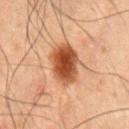notes = imaged on a skin check; not biopsied
image-analysis metrics = a footprint of about 14 mm², an eccentricity of roughly 0.7, and a symmetry-axis asymmetry near 0.15; border irregularity of about 1.5 on a 0–10 scale, a within-lesion color-variation index near 5.5/10, and peripheral color asymmetry of about 1.5; a classifier nevus-likeness of about 100/100 and lesion-presence confidence of about 100/100
subject = male, aged 48–52
anatomic site = the abdomen
imaging modality = 15 mm crop, total-body photography
lighting = cross-polarized illumination
diameter = ~5 mm (longest diameter)